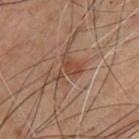Impression: Imaged during a routine full-body skin examination; the lesion was not biopsied and no histopathology is available. Acquisition and patient details: The recorded lesion diameter is about 3.5 mm. A 15 mm close-up tile from a total-body photography series done for melanoma screening. The patient is a male in their mid-50s. The lesion is on the front of the torso.